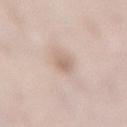Background:
A region of skin cropped from a whole-body photographic capture, roughly 15 mm wide. A female patient aged around 50. The lesion is located on the back. Captured under white-light illumination.